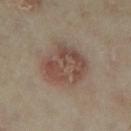Clinical summary: A female patient, aged approximately 35. A roughly 15 mm field-of-view crop from a total-body skin photograph. The lesion is on the right lower leg.